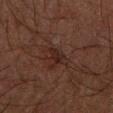biopsy status = no biopsy performed (imaged during a skin exam)
patient = male, aged 48 to 52
image source = total-body-photography crop, ~15 mm field of view
anatomic site = the right forearm